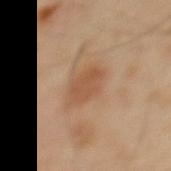Imaged during a routine full-body skin examination; the lesion was not biopsied and no histopathology is available.
Imaged with cross-polarized lighting.
The lesion is on the mid back.
A 15 mm crop from a total-body photograph taken for skin-cancer surveillance.
A male patient aged approximately 40.
The lesion's longest dimension is about 4 mm.